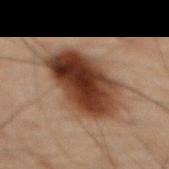Impression: Captured during whole-body skin photography for melanoma surveillance; the lesion was not biopsied. Clinical summary: The lesion's longest dimension is about 7 mm. A 15 mm close-up tile from a total-body photography series done for melanoma screening. The total-body-photography lesion software estimated an area of roughly 33 mm², an outline eccentricity of about 0.65 (0 = round, 1 = elongated), and a symmetry-axis asymmetry near 0.15. And it measured a mean CIELAB color near L≈29 a*≈16 b*≈23, about 14 CIELAB-L* units darker than the surrounding skin, and a normalized border contrast of about 13.5. It also reported internal color variation of about 8 on a 0–10 scale and peripheral color asymmetry of about 2.5. It also reported a nevus-likeness score of about 100/100 and a lesion-detection confidence of about 100/100. The lesion is on the front of the torso. The tile uses cross-polarized illumination. The patient is a male aged 68–72.